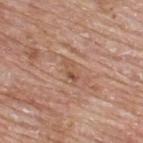Impression: No biopsy was performed on this lesion — it was imaged during a full skin examination and was not determined to be concerning. Context: The lesion is located on the upper back. A roughly 15 mm field-of-view crop from a total-body skin photograph. A male patient aged around 65.